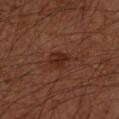subject: male, approximately 60 years of age
illumination: cross-polarized illumination
lesion diameter: about 2.5 mm
site: the right forearm
image source: 15 mm crop, total-body photography